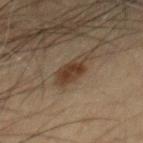Clinical impression:
This lesion was catalogued during total-body skin photography and was not selected for biopsy.
Image and clinical context:
Cropped from a whole-body photographic skin survey; the tile spans about 15 mm. An algorithmic analysis of the crop reported an area of roughly 7 mm², an outline eccentricity of about 0.85 (0 = round, 1 = elongated), and a symmetry-axis asymmetry near 0.15. It also reported an average lesion color of about L≈32 a*≈13 b*≈24 (CIELAB), roughly 9 lightness units darker than nearby skin, and a normalized border contrast of about 9. The analysis additionally found border irregularity of about 2 on a 0–10 scale, a within-lesion color-variation index near 3.5/10, and radial color variation of about 1. And it measured a nevus-likeness score of about 95/100 and a lesion-detection confidence of about 100/100. A male patient roughly 60 years of age. This is a cross-polarized tile. The lesion's longest dimension is about 4 mm.